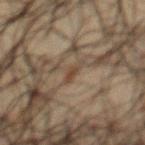Q: Was this lesion biopsied?
A: total-body-photography surveillance lesion; no biopsy
Q: Lesion location?
A: the chest
Q: What is the imaging modality?
A: 15 mm crop, total-body photography
Q: What are the patient's age and sex?
A: male, in their 60s